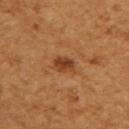Q: Is there a histopathology result?
A: imaged on a skin check; not biopsied
Q: What are the patient's age and sex?
A: female, aged around 55
Q: How was the tile lit?
A: cross-polarized
Q: What is the imaging modality?
A: ~15 mm crop, total-body skin-cancer survey
Q: What is the anatomic site?
A: the right upper arm